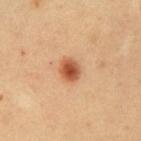Clinical impression: Captured during whole-body skin photography for melanoma surveillance; the lesion was not biopsied. Background: Imaged with cross-polarized lighting. A male patient, aged 48–52. On the abdomen. A lesion tile, about 15 mm wide, cut from a 3D total-body photograph. The total-body-photography lesion software estimated a classifier nevus-likeness of about 100/100. The lesion's longest dimension is about 2.5 mm.